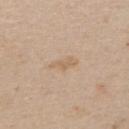Acquisition and patient details: The total-body-photography lesion software estimated a border-irregularity index near 3.5/10, a within-lesion color-variation index near 1.5/10, and a peripheral color-asymmetry measure near 0.5. About 3.5 mm across. Located on the back. A lesion tile, about 15 mm wide, cut from a 3D total-body photograph. A female patient about 45 years old.